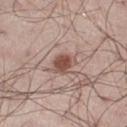A 15 mm close-up tile from a total-body photography series done for melanoma screening.
Automated image analysis of the tile measured an automated nevus-likeness rating near 95 out of 100 and lesion-presence confidence of about 100/100.
A male subject, in their 50s.
The lesion is on the right thigh.